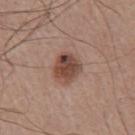Imaged with white-light lighting. On the chest. The subject is a male roughly 75 years of age. A 15 mm close-up tile from a total-body photography series done for melanoma screening. About 4 mm across.